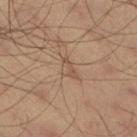| field | value |
|---|---|
| workup | no biopsy performed (imaged during a skin exam) |
| TBP lesion metrics | an average lesion color of about L≈47 a*≈16 b*≈26 (CIELAB) and a lesion-to-skin contrast of about 5 (normalized; higher = more distinct); a border-irregularity rating of about 4/10, internal color variation of about 1.5 on a 0–10 scale, and a peripheral color-asymmetry measure near 0.5; an automated nevus-likeness rating near 0 out of 100 and lesion-presence confidence of about 90/100 |
| tile lighting | cross-polarized |
| image source | 15 mm crop, total-body photography |
| site | the leg |
| subject | male, about 35 years old |